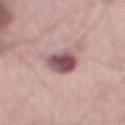No biopsy was performed on this lesion — it was imaged during a full skin examination and was not determined to be concerning. The lesion is located on the mid back. Approximately 4 mm at its widest. This is a white-light tile. A male subject approximately 65 years of age. Cropped from a whole-body photographic skin survey; the tile spans about 15 mm.